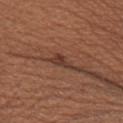Findings:
– notes · imaged on a skin check; not biopsied
– anatomic site · the right upper arm
– diameter · ≈3 mm
– image · 15 mm crop, total-body photography
– patient · female, aged 43 to 47
– lighting · white-light